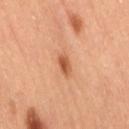Captured during whole-body skin photography for melanoma surveillance; the lesion was not biopsied.
About 2.5 mm across.
Cropped from a total-body skin-imaging series; the visible field is about 15 mm.
The subject is a female in their 60s.
Automated image analysis of the tile measured a shape eccentricity near 0.85 and two-axis asymmetry of about 0.25. It also reported a lesion color around L≈58 a*≈28 b*≈39 in CIELAB and a lesion–skin lightness drop of about 13. It also reported a lesion-detection confidence of about 100/100.
On the lower back.